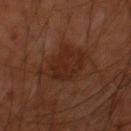Part of a total-body skin-imaging series; this lesion was reviewed on a skin check and was not flagged for biopsy. Located on the right thigh. Cropped from a total-body skin-imaging series; the visible field is about 15 mm. Imaged with cross-polarized lighting. Approximately 5.5 mm at its widest. The subject is a male aged approximately 65. The total-body-photography lesion software estimated an area of roughly 15 mm² and an eccentricity of roughly 0.7. The software also gave border irregularity of about 4 on a 0–10 scale, a color-variation rating of about 3/10, and radial color variation of about 1. The analysis additionally found an automated nevus-likeness rating near 50 out of 100.